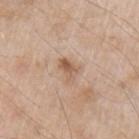Imaged during a routine full-body skin examination; the lesion was not biopsied and no histopathology is available. The lesion's longest dimension is about 3 mm. This image is a 15 mm lesion crop taken from a total-body photograph. The subject is a male in their mid- to late 60s. This is a white-light tile. On the arm.